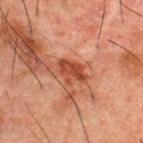The lesion was tiled from a total-body skin photograph and was not biopsied. Cropped from a total-body skin-imaging series; the visible field is about 15 mm. From the upper back. Imaged with cross-polarized lighting. A male patient, aged around 50. Longest diameter approximately 3.5 mm.